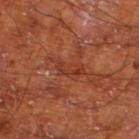workup = imaged on a skin check; not biopsied | body site = the left lower leg | image = total-body-photography crop, ~15 mm field of view | diameter = ≈3.5 mm | tile lighting = cross-polarized | patient = male, in their mid-60s.